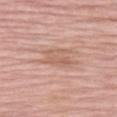The lesion was photographed on a routine skin check and not biopsied; there is no pathology result. The subject is a female aged around 70. Approximately 4.5 mm at its widest. This image is a 15 mm lesion crop taken from a total-body photograph. This is a white-light tile. Automated image analysis of the tile measured a lesion color around L≈61 a*≈21 b*≈28 in CIELAB and a normalized lesion–skin contrast near 5. And it measured border irregularity of about 5 on a 0–10 scale, a within-lesion color-variation index near 3.5/10, and a peripheral color-asymmetry measure near 1. The software also gave an automated nevus-likeness rating near 0 out of 100 and a lesion-detection confidence of about 100/100. Located on the left thigh.